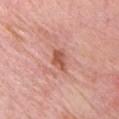{
  "biopsy_status": "not biopsied; imaged during a skin examination",
  "site": "chest",
  "automated_metrics": {
    "area_mm2_approx": 4.5,
    "eccentricity": 0.65,
    "shape_asymmetry": 0.3,
    "cielab_L": 56,
    "cielab_a": 27,
    "cielab_b": 31,
    "vs_skin_darker_L": 11.0,
    "vs_skin_contrast_norm": 7.5
  },
  "patient": {
    "sex": "male",
    "age_approx": 65
  },
  "image": {
    "source": "total-body photography crop",
    "field_of_view_mm": 15
  },
  "lesion_size": {
    "long_diameter_mm_approx": 3.0
  }
}